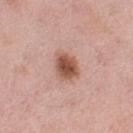Assessment: No biopsy was performed on this lesion — it was imaged during a full skin examination and was not determined to be concerning. Acquisition and patient details: On the left thigh. A female patient, aged approximately 50. A region of skin cropped from a whole-body photographic capture, roughly 15 mm wide.